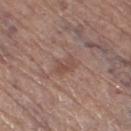| feature | finding |
|---|---|
| biopsy status | total-body-photography surveillance lesion; no biopsy |
| subject | male, in their mid-60s |
| size | ~3 mm (longest diameter) |
| lighting | white-light |
| automated lesion analysis | a border-irregularity index near 2.5/10, internal color variation of about 3 on a 0–10 scale, and radial color variation of about 1 |
| body site | the right thigh |
| acquisition | ~15 mm tile from a whole-body skin photo |